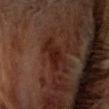<tbp_lesion>
  <biopsy_status>not biopsied; imaged during a skin examination</biopsy_status>
  <lesion_size>
    <long_diameter_mm_approx>5.0</long_diameter_mm_approx>
  </lesion_size>
  <site>head or neck</site>
  <image>
    <source>total-body photography crop</source>
    <field_of_view_mm>15</field_of_view_mm>
  </image>
  <lighting>cross-polarized</lighting>
  <patient>
    <sex>male</sex>
    <age_approx>65</age_approx>
  </patient>
</tbp_lesion>